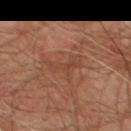Imaged during a routine full-body skin examination; the lesion was not biopsied and no histopathology is available. The lesion is located on the front of the torso. This is a cross-polarized tile. The recorded lesion diameter is about 4.5 mm. The total-body-photography lesion software estimated a footprint of about 6 mm², an outline eccentricity of about 0.9 (0 = round, 1 = elongated), and two-axis asymmetry of about 0.55. And it measured an average lesion color of about L≈35 a*≈18 b*≈25 (CIELAB), about 5 CIELAB-L* units darker than the surrounding skin, and a normalized border contrast of about 5. A male subject aged approximately 75. A close-up tile cropped from a whole-body skin photograph, about 15 mm across.